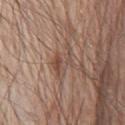Part of a total-body skin-imaging series; this lesion was reviewed on a skin check and was not flagged for biopsy.
The subject is a male aged 78 to 82.
On the chest.
Imaged with white-light lighting.
An algorithmic analysis of the crop reported a mean CIELAB color near L≈48 a*≈17 b*≈25, about 8 CIELAB-L* units darker than the surrounding skin, and a lesion-to-skin contrast of about 6 (normalized; higher = more distinct). The software also gave a border-irregularity index near 7/10, internal color variation of about 4.5 on a 0–10 scale, and peripheral color asymmetry of about 1.5.
The lesion's longest dimension is about 4 mm.
A close-up tile cropped from a whole-body skin photograph, about 15 mm across.